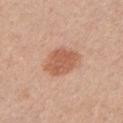The lesion was tiled from a total-body skin photograph and was not biopsied.
Measured at roughly 5 mm in maximum diameter.
A male subject aged 48–52.
This is a white-light tile.
Cropped from a total-body skin-imaging series; the visible field is about 15 mm.
The lesion is on the chest.